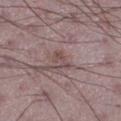The lesion was photographed on a routine skin check and not biopsied; there is no pathology result.